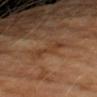Clinical impression:
The lesion was photographed on a routine skin check and not biopsied; there is no pathology result.